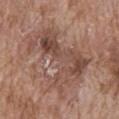| feature | finding |
|---|---|
| follow-up | no biopsy performed (imaged during a skin exam) |
| anatomic site | the front of the torso |
| TBP lesion metrics | a footprint of about 33 mm² and a shape-asymmetry score of about 0.55 (0 = symmetric); a within-lesion color-variation index near 6.5/10 and a peripheral color-asymmetry measure near 2.5; an automated nevus-likeness rating near 0 out of 100 and a detector confidence of about 100 out of 100 that the crop contains a lesion |
| image | ~15 mm crop, total-body skin-cancer survey |
| patient | male, aged 73 to 77 |
| diameter | ≈9 mm |
| illumination | white-light illumination |